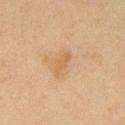The lesion was tiled from a total-body skin photograph and was not biopsied. Approximately 2.5 mm at its widest. A 15 mm crop from a total-body photograph taken for skin-cancer surveillance. A female patient in their 40s. Captured under cross-polarized illumination. From the chest.